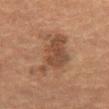<record>
<biopsy_status>not biopsied; imaged during a skin examination</biopsy_status>
<lesion_size>
  <long_diameter_mm_approx>5.5</long_diameter_mm_approx>
</lesion_size>
<site>abdomen</site>
<image>
  <source>total-body photography crop</source>
  <field_of_view_mm>15</field_of_view_mm>
</image>
<patient>
  <sex>female</sex>
  <age_approx>70</age_approx>
</patient>
<automated_metrics>
  <eccentricity>0.75</eccentricity>
  <cielab_L>39</cielab_L>
  <cielab_a>17</cielab_a>
  <cielab_b>27</cielab_b>
  <vs_skin_darker_L>8.0</vs_skin_darker_L>
  <vs_skin_contrast_norm>7.0</vs_skin_contrast_norm>
  <nevus_likeness_0_100>35</nevus_likeness_0_100>
  <lesion_detection_confidence_0_100>100</lesion_detection_confidence_0_100>
</automated_metrics>
</record>